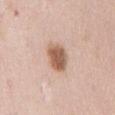  biopsy_status: not biopsied; imaged during a skin examination
  site: abdomen
  lighting: white-light
  patient:
    sex: female
    age_approx: 65
  image:
    source: total-body photography crop
    field_of_view_mm: 15